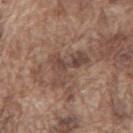workup — total-body-photography surveillance lesion; no biopsy.